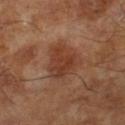Q: Was a biopsy performed?
A: catalogued during a skin exam; not biopsied
Q: Who is the patient?
A: male, aged approximately 65
Q: How was this image acquired?
A: ~15 mm crop, total-body skin-cancer survey
Q: What lighting was used for the tile?
A: cross-polarized illumination